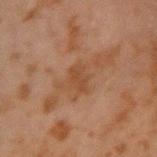  biopsy_status: not biopsied; imaged during a skin examination
  image:
    source: total-body photography crop
    field_of_view_mm: 15
  site: right upper arm
  patient:
    sex: male
    age_approx: 45
  lesion_size:
    long_diameter_mm_approx: 2.5
  lighting: cross-polarized